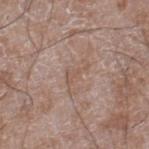Clinical impression: The lesion was tiled from a total-body skin photograph and was not biopsied. Context: On the right lower leg. The tile uses white-light illumination. A roughly 15 mm field-of-view crop from a total-body skin photograph. A male subject roughly 60 years of age.